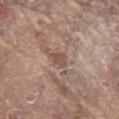The lesion was photographed on a routine skin check and not biopsied; there is no pathology result. Longest diameter approximately 2.5 mm. Imaged with white-light lighting. The lesion is on the abdomen. A male subject about 80 years old. Automated tile analysis of the lesion measured an outline eccentricity of about 0.7 (0 = round, 1 = elongated). It also reported border irregularity of about 3.5 on a 0–10 scale, internal color variation of about 2 on a 0–10 scale, and peripheral color asymmetry of about 0.5. This image is a 15 mm lesion crop taken from a total-body photograph.